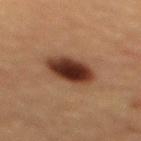The lesion was photographed on a routine skin check and not biopsied; there is no pathology result.
This is a cross-polarized tile.
A 15 mm close-up extracted from a 3D total-body photography capture.
The subject is a female aged 68–72.
From the mid back.